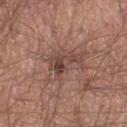Recorded during total-body skin imaging; not selected for excision or biopsy. The recorded lesion diameter is about 6 mm. The subject is a male about 40 years old. Cropped from a total-body skin-imaging series; the visible field is about 15 mm. The lesion is on the leg. This is a white-light tile.